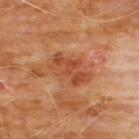The lesion was tiled from a total-body skin photograph and was not biopsied. The lesion is located on the front of the torso. This is a cross-polarized tile. The patient is a male approximately 60 years of age. Cropped from a whole-body photographic skin survey; the tile spans about 15 mm.